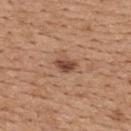Image and clinical context: On the upper back. The subject is a female about 45 years old. A region of skin cropped from a whole-body photographic capture, roughly 15 mm wide. Imaged with white-light lighting. About 3 mm across.